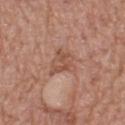subject=male, aged approximately 75
tile lighting=white-light illumination
site=the upper back
automated lesion analysis=a lesion area of about 7 mm² and two-axis asymmetry of about 0.4; a nevus-likeness score of about 0/100 and a detector confidence of about 100 out of 100 that the crop contains a lesion
imaging modality=~15 mm tile from a whole-body skin photo